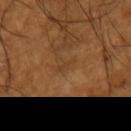Background: Imaged with cross-polarized lighting. A 15 mm close-up tile from a total-body photography series done for melanoma screening. The subject is a male aged approximately 65. The lesion's longest dimension is about 2.5 mm. From the left upper arm.